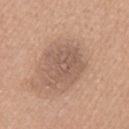Clinical impression: No biopsy was performed on this lesion — it was imaged during a full skin examination and was not determined to be concerning. Image and clinical context: A roughly 15 mm field-of-view crop from a total-body skin photograph. A female subject, aged 53 to 57. The lesion is on the mid back. This is a white-light tile. About 4.5 mm across.